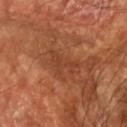biopsy_status: not biopsied; imaged during a skin examination